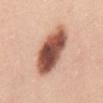| feature | finding |
|---|---|
| workup | imaged on a skin check; not biopsied |
| lighting | white-light |
| subject | female, approximately 30 years of age |
| site | the chest |
| image | total-body-photography crop, ~15 mm field of view |